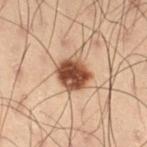Imaged during a routine full-body skin examination; the lesion was not biopsied and no histopathology is available. Captured under cross-polarized illumination. A male subject, aged 53–57. A 15 mm close-up extracted from a 3D total-body photography capture. The recorded lesion diameter is about 3.5 mm. Located on the right thigh.